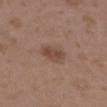The lesion was tiled from a total-body skin photograph and was not biopsied. A 15 mm crop from a total-body photograph taken for skin-cancer surveillance. About 3.5 mm across. The lesion is located on the upper back. This is a white-light tile. The subject is a female in their mid-30s.